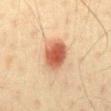Captured during whole-body skin photography for melanoma surveillance; the lesion was not biopsied. Cropped from a whole-body photographic skin survey; the tile spans about 15 mm. A male subject, aged approximately 40. This is a cross-polarized tile. Located on the abdomen. Automated image analysis of the tile measured an outline eccentricity of about 0.5 (0 = round, 1 = elongated) and two-axis asymmetry of about 0.15. The analysis additionally found a mean CIELAB color near L≈53 a*≈25 b*≈32 and a lesion-to-skin contrast of about 10 (normalized; higher = more distinct). The analysis additionally found border irregularity of about 1.5 on a 0–10 scale and radial color variation of about 1. It also reported an automated nevus-likeness rating near 100 out of 100 and a lesion-detection confidence of about 100/100. Measured at roughly 4 mm in maximum diameter.